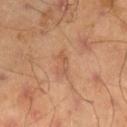| key | value |
|---|---|
| biopsy status | catalogued during a skin exam; not biopsied |
| tile lighting | cross-polarized |
| imaging modality | total-body-photography crop, ~15 mm field of view |
| body site | the leg |
| TBP lesion metrics | a footprint of about 2.5 mm²; a mean CIELAB color near L≈54 a*≈22 b*≈34 and a lesion–skin lightness drop of about 7; a within-lesion color-variation index near 0/10; an automated nevus-likeness rating near 0 out of 100 and a lesion-detection confidence of about 100/100 |
| lesion diameter | ~2.5 mm (longest diameter) |
| patient | male, roughly 45 years of age |